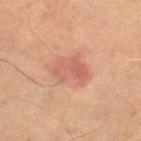Assessment: The lesion was photographed on a routine skin check and not biopsied; there is no pathology result. Context: Automated tile analysis of the lesion measured an area of roughly 9 mm² and two-axis asymmetry of about 0.35. It also reported a within-lesion color-variation index near 4/10. A 15 mm crop from a total-body photograph taken for skin-cancer surveillance. A male subject, aged 68–72. The lesion's longest dimension is about 4 mm. This is a cross-polarized tile. From the right thigh.